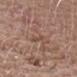Captured during whole-body skin photography for melanoma surveillance; the lesion was not biopsied.
A male subject, approximately 80 years of age.
A 15 mm close-up tile from a total-body photography series done for melanoma screening.
The tile uses white-light illumination.
Automated tile analysis of the lesion measured a lesion color around L≈48 a*≈19 b*≈26 in CIELAB, a lesion–skin lightness drop of about 7, and a normalized border contrast of about 5.5. The analysis additionally found a nevus-likeness score of about 0/100 and a lesion-detection confidence of about 65/100.
Longest diameter approximately 3.5 mm.
On the chest.